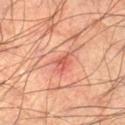Impression: The lesion was tiled from a total-body skin photograph and was not biopsied. Background: A male subject aged around 45. Cropped from a total-body skin-imaging series; the visible field is about 15 mm. The tile uses cross-polarized illumination. Automated tile analysis of the lesion measured an area of roughly 3.5 mm², an outline eccentricity of about 0.85 (0 = round, 1 = elongated), and a symmetry-axis asymmetry near 0.25. It also reported border irregularity of about 2.5 on a 0–10 scale, internal color variation of about 2.5 on a 0–10 scale, and radial color variation of about 0.5. And it measured a classifier nevus-likeness of about 0/100. On the right lower leg.